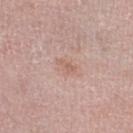| field | value |
|---|---|
| workup | no biopsy performed (imaged during a skin exam) |
| body site | the right lower leg |
| imaging modality | 15 mm crop, total-body photography |
| subject | female, about 55 years old |